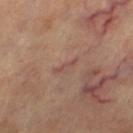Captured during whole-body skin photography for melanoma surveillance; the lesion was not biopsied.
Imaged with cross-polarized lighting.
About 3 mm across.
A female subject about 60 years old.
From the right leg.
A lesion tile, about 15 mm wide, cut from a 3D total-body photograph.